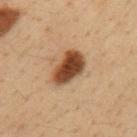Findings:
• biopsy status: total-body-photography surveillance lesion; no biopsy
• automated metrics: a border-irregularity index near 2/10, a color-variation rating of about 5/10, and peripheral color asymmetry of about 1.5; an automated nevus-likeness rating near 100 out of 100 and lesion-presence confidence of about 100/100
• lighting: cross-polarized
• lesion size: ≈5 mm
• site: the left upper arm
• image: ~15 mm tile from a whole-body skin photo
• patient: male, roughly 35 years of age